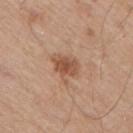follow-up=total-body-photography surveillance lesion; no biopsy
image source=total-body-photography crop, ~15 mm field of view
lesion diameter=~4 mm (longest diameter)
patient=male, aged 53 to 57
location=the right upper arm
lighting=white-light